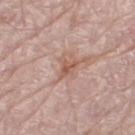The lesion was photographed on a routine skin check and not biopsied; there is no pathology result. A close-up tile cropped from a whole-body skin photograph, about 15 mm across. Imaged with white-light lighting. Located on the left thigh. Longest diameter approximately 3 mm. The patient is a female in their mid-60s.